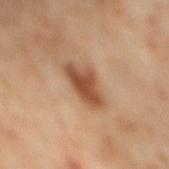The tile uses cross-polarized illumination.
A male patient aged around 60.
From the mid back.
This image is a 15 mm lesion crop taken from a total-body photograph.
Automated tile analysis of the lesion measured a shape eccentricity near 0.85 and a symmetry-axis asymmetry near 0.25. The software also gave a nevus-likeness score of about 90/100 and lesion-presence confidence of about 100/100.
The recorded lesion diameter is about 4.5 mm.